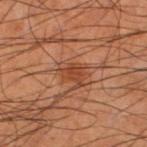Findings:
– biopsy status: no biopsy performed (imaged during a skin exam)
– acquisition: ~15 mm tile from a whole-body skin photo
– subject: male, approximately 65 years of age
– anatomic site: the left lower leg